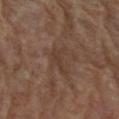Imaged during a routine full-body skin examination; the lesion was not biopsied and no histopathology is available. A 15 mm close-up tile from a total-body photography series done for melanoma screening. The lesion is located on the left forearm. The patient is a male aged 83 or older.